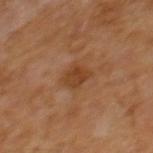Q: Was this lesion biopsied?
A: total-body-photography surveillance lesion; no biopsy
Q: How was the tile lit?
A: cross-polarized illumination
Q: What are the patient's age and sex?
A: male, about 65 years old
Q: What kind of image is this?
A: ~15 mm tile from a whole-body skin photo
Q: Automated lesion metrics?
A: a lesion area of about 4.5 mm², an outline eccentricity of about 0.55 (0 = round, 1 = elongated), and a shape-asymmetry score of about 0.25 (0 = symmetric)
Q: How large is the lesion?
A: about 2.5 mm
Q: What is the anatomic site?
A: the back